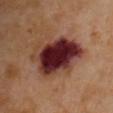Assessment: This lesion was catalogued during total-body skin photography and was not selected for biopsy. Background: A 15 mm crop from a total-body photograph taken for skin-cancer surveillance. A female patient, roughly 55 years of age. Located on the chest. An algorithmic analysis of the crop reported an automated nevus-likeness rating near 5 out of 100 and a lesion-detection confidence of about 100/100. Captured under cross-polarized illumination. Longest diameter approximately 7 mm.